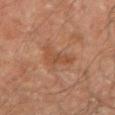Assessment:
Part of a total-body skin-imaging series; this lesion was reviewed on a skin check and was not flagged for biopsy.